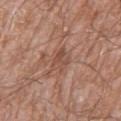– notes — catalogued during a skin exam; not biopsied
– subject — male, aged around 60
– size — about 3.5 mm
– imaging modality — total-body-photography crop, ~15 mm field of view
– location — the right thigh
– image-analysis metrics — a lesion color around L≈50 a*≈21 b*≈28 in CIELAB, a lesion–skin lightness drop of about 7, and a lesion-to-skin contrast of about 5.5 (normalized; higher = more distinct); a lesion-detection confidence of about 95/100